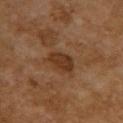workup: total-body-photography surveillance lesion; no biopsy | lighting: cross-polarized | lesion size: ~3.5 mm (longest diameter) | image: ~15 mm tile from a whole-body skin photo | patient: female, aged 58–62 | anatomic site: the upper back.